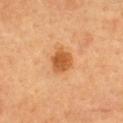Impression: Part of a total-body skin-imaging series; this lesion was reviewed on a skin check and was not flagged for biopsy. Background: A female patient, roughly 60 years of age. Cropped from a whole-body photographic skin survey; the tile spans about 15 mm. Automated image analysis of the tile measured about 11 CIELAB-L* units darker than the surrounding skin and a lesion-to-skin contrast of about 8.5 (normalized; higher = more distinct). On the upper back. Imaged with cross-polarized lighting.